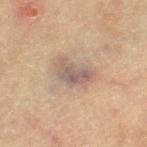Part of a total-body skin-imaging series; this lesion was reviewed on a skin check and was not flagged for biopsy.
Cropped from a whole-body photographic skin survey; the tile spans about 15 mm.
A female patient aged 78 to 82.
From the left thigh.